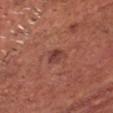The lesion was photographed on a routine skin check and not biopsied; there is no pathology result.
The subject is a male in their mid-60s.
On the head or neck.
Cropped from a whole-body photographic skin survey; the tile spans about 15 mm.